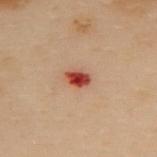Q: Was this lesion biopsied?
A: imaged on a skin check; not biopsied
Q: Who is the patient?
A: female, roughly 60 years of age
Q: Illumination type?
A: cross-polarized
Q: What did automated image analysis measure?
A: a mean CIELAB color near L≈41 a*≈30 b*≈29, about 15 CIELAB-L* units darker than the surrounding skin, and a lesion-to-skin contrast of about 12 (normalized; higher = more distinct); a nevus-likeness score of about 0/100
Q: What is the imaging modality?
A: ~15 mm tile from a whole-body skin photo
Q: What is the lesion's diameter?
A: ~3 mm (longest diameter)
Q: Where on the body is the lesion?
A: the upper back